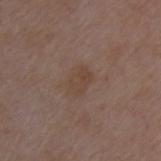Clinical summary: About 3 mm across. This is a white-light tile. A region of skin cropped from a whole-body photographic capture, roughly 15 mm wide. Located on the chest. The subject is a male aged approximately 50.